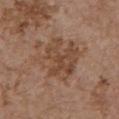Image and clinical context:
Located on the chest. The recorded lesion diameter is about 5.5 mm. A close-up tile cropped from a whole-body skin photograph, about 15 mm across. The tile uses white-light illumination. The patient is a female aged around 65. The total-body-photography lesion software estimated border irregularity of about 4 on a 0–10 scale, a within-lesion color-variation index near 4.5/10, and a peripheral color-asymmetry measure near 1.5.